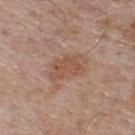On the upper back.
Captured under white-light illumination.
The recorded lesion diameter is about 4.5 mm.
A male subject, aged approximately 55.
A lesion tile, about 15 mm wide, cut from a 3D total-body photograph.